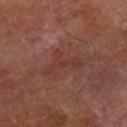Notes:
• follow-up — imaged on a skin check; not biopsied
• site — the right forearm
• subject — male, aged around 70
• imaging modality — ~15 mm tile from a whole-body skin photo